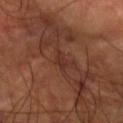workup=no biopsy performed (imaged during a skin exam)
automated metrics=about 5 CIELAB-L* units darker than the surrounding skin and a normalized border contrast of about 5.5
subject=male, approximately 55 years of age
lesion diameter=~2.5 mm (longest diameter)
illumination=cross-polarized illumination
acquisition=~15 mm tile from a whole-body skin photo
body site=the right forearm